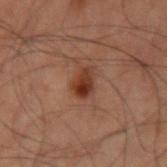This lesion was catalogued during total-body skin photography and was not selected for biopsy. A male subject roughly 60 years of age. A 15 mm crop from a total-body photograph taken for skin-cancer surveillance. The total-body-photography lesion software estimated border irregularity of about 2.5 on a 0–10 scale, internal color variation of about 5.5 on a 0–10 scale, and radial color variation of about 2. Longest diameter approximately 3 mm. Located on the left upper arm.